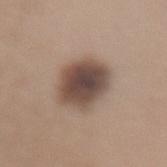notes: total-body-photography surveillance lesion; no biopsy | lighting: white-light illumination | diameter: about 5 mm | imaging modality: 15 mm crop, total-body photography | patient: female, in their 40s | automated lesion analysis: a lesion area of about 17 mm², a shape eccentricity near 0.35, and two-axis asymmetry of about 0.15; a border-irregularity index near 1.5/10, a within-lesion color-variation index near 5.5/10, and peripheral color asymmetry of about 1.5 | location: the lower back.